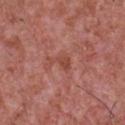<tbp_lesion>
<biopsy_status>not biopsied; imaged during a skin examination</biopsy_status>
<image>
  <source>total-body photography crop</source>
  <field_of_view_mm>15</field_of_view_mm>
</image>
<automated_metrics>
  <area_mm2_approx>3.0</area_mm2_approx>
  <eccentricity>0.75</eccentricity>
  <shape_asymmetry>0.6</shape_asymmetry>
  <border_irregularity_0_10>6.0</border_irregularity_0_10>
  <peripheral_color_asymmetry>0.5</peripheral_color_asymmetry>
</automated_metrics>
<site>chest</site>
<patient>
  <sex>male</sex>
  <age_approx>45</age_approx>
</patient>
<lesion_size>
  <long_diameter_mm_approx>2.5</long_diameter_mm_approx>
</lesion_size>
<lighting>white-light</lighting>
</tbp_lesion>